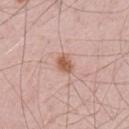Findings:
* follow-up — total-body-photography surveillance lesion; no biopsy
* acquisition — ~15 mm tile from a whole-body skin photo
* subject — male, aged approximately 55
* site — the left thigh